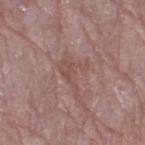Q: Was this lesion biopsied?
A: imaged on a skin check; not biopsied
Q: What lighting was used for the tile?
A: white-light
Q: How was this image acquired?
A: 15 mm crop, total-body photography
Q: Where on the body is the lesion?
A: the leg
Q: What are the patient's age and sex?
A: female, about 65 years old
Q: Lesion size?
A: ≈5.5 mm